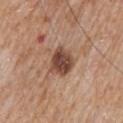Q: What lighting was used for the tile?
A: white-light
Q: Who is the patient?
A: male, about 60 years old
Q: What is the imaging modality?
A: ~15 mm crop, total-body skin-cancer survey
Q: What did automated image analysis measure?
A: a classifier nevus-likeness of about 55/100 and a detector confidence of about 100 out of 100 that the crop contains a lesion
Q: Where on the body is the lesion?
A: the mid back
Q: What is the lesion's diameter?
A: ~3.5 mm (longest diameter)